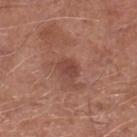No biopsy was performed on this lesion — it was imaged during a full skin examination and was not determined to be concerning. The lesion is located on the left lower leg. A male subject, in their mid-60s. Captured under white-light illumination. A 15 mm close-up tile from a total-body photography series done for melanoma screening.